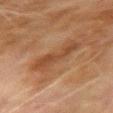biopsy status: total-body-photography surveillance lesion; no biopsy | image: total-body-photography crop, ~15 mm field of view | lighting: cross-polarized illumination | anatomic site: the arm | subject: male, aged approximately 70 | lesion size: ~4.5 mm (longest diameter).